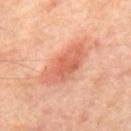follow-up = total-body-photography surveillance lesion; no biopsy
automated metrics = a mean CIELAB color near L≈60 a*≈27 b*≈34 and a normalized lesion–skin contrast near 6.5; border irregularity of about 3.5 on a 0–10 scale and peripheral color asymmetry of about 1
lesion diameter = about 6.5 mm
imaging modality = 15 mm crop, total-body photography
tile lighting = cross-polarized
body site = the mid back
patient = male, aged 63 to 67